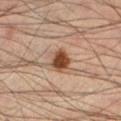Impression:
Captured during whole-body skin photography for melanoma surveillance; the lesion was not biopsied.
Image and clinical context:
A male subject, approximately 35 years of age. The lesion's longest dimension is about 3 mm. On the left lower leg. Captured under cross-polarized illumination. The total-body-photography lesion software estimated an area of roughly 5.5 mm², an eccentricity of roughly 0.45, and a symmetry-axis asymmetry near 0.2. The analysis additionally found roughly 16 lightness units darker than nearby skin. And it measured a border-irregularity rating of about 2/10 and peripheral color asymmetry of about 1. The software also gave an automated nevus-likeness rating near 100 out of 100 and lesion-presence confidence of about 100/100. Cropped from a whole-body photographic skin survey; the tile spans about 15 mm.